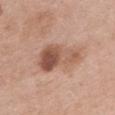Impression: Part of a total-body skin-imaging series; this lesion was reviewed on a skin check and was not flagged for biopsy. Context: The patient is a female roughly 60 years of age. Approximately 5.5 mm at its widest. A region of skin cropped from a whole-body photographic capture, roughly 15 mm wide. The lesion is on the front of the torso. Imaged with white-light lighting.